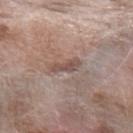Impression: Captured during whole-body skin photography for melanoma surveillance; the lesion was not biopsied. Context: This is a white-light tile. Longest diameter approximately 3.5 mm. A 15 mm close-up extracted from a 3D total-body photography capture. The patient is a female roughly 75 years of age. The lesion is located on the left forearm.